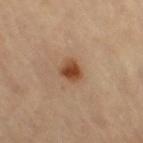follow-up — catalogued during a skin exam; not biopsied
image source — ~15 mm crop, total-body skin-cancer survey
illumination — cross-polarized
location — the leg
subject — female, aged 68–72